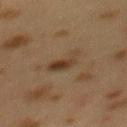Notes:
• biopsy status · imaged on a skin check; not biopsied
• subject · female, aged 38 to 42
• acquisition · ~15 mm tile from a whole-body skin photo
• illumination · cross-polarized
• location · the back
• automated metrics · a color-variation rating of about 4/10